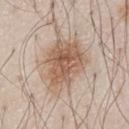Recorded during total-body skin imaging; not selected for excision or biopsy. Imaged with white-light lighting. The subject is a male aged 78–82. The total-body-photography lesion software estimated border irregularity of about 3.5 on a 0–10 scale, a within-lesion color-variation index near 5/10, and a peripheral color-asymmetry measure near 1.5. The lesion is located on the chest. Longest diameter approximately 6.5 mm. Cropped from a whole-body photographic skin survey; the tile spans about 15 mm.